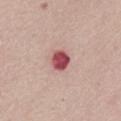Clinical impression: No biopsy was performed on this lesion — it was imaged during a full skin examination and was not determined to be concerning. Background: The patient is a female roughly 65 years of age. This is a white-light tile. A 15 mm close-up extracted from a 3D total-body photography capture. Automated tile analysis of the lesion measured a lesion area of about 6 mm², an eccentricity of roughly 0.2, and two-axis asymmetry of about 0.15. The software also gave a lesion color around L≈52 a*≈31 b*≈21 in CIELAB and a normalized lesion–skin contrast near 11.5. And it measured an automated nevus-likeness rating near 0 out of 100 and a lesion-detection confidence of about 100/100. The lesion is on the front of the torso. Longest diameter approximately 2.5 mm.